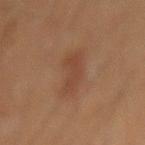Q: Is there a histopathology result?
A: catalogued during a skin exam; not biopsied
Q: Who is the patient?
A: male, approximately 60 years of age
Q: What is the anatomic site?
A: the abdomen
Q: Lesion size?
A: about 5 mm
Q: What did automated image analysis measure?
A: an area of roughly 9.5 mm², an eccentricity of roughly 0.9, and a shape-asymmetry score of about 0.3 (0 = symmetric); a lesion–skin lightness drop of about 6 and a normalized border contrast of about 5; a border-irregularity rating of about 3.5/10, a within-lesion color-variation index near 2/10, and radial color variation of about 0.5; an automated nevus-likeness rating near 10 out of 100
Q: How was this image acquired?
A: ~15 mm crop, total-body skin-cancer survey